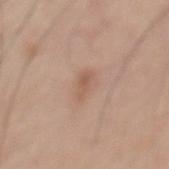follow-up = no biopsy performed (imaged during a skin exam)
image = total-body-photography crop, ~15 mm field of view
lesion diameter = about 2.5 mm
TBP lesion metrics = an area of roughly 2.5 mm², a shape eccentricity near 0.9, and two-axis asymmetry of about 0.35; border irregularity of about 3.5 on a 0–10 scale, a color-variation rating of about 0.5/10, and peripheral color asymmetry of about 0; an automated nevus-likeness rating near 5 out of 100 and lesion-presence confidence of about 100/100
site = the mid back
illumination = white-light illumination
subject = male, roughly 65 years of age